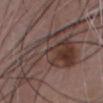biopsy status=total-body-photography surveillance lesion; no biopsy
location=the chest
lighting=white-light illumination
image=total-body-photography crop, ~15 mm field of view
subject=male, approximately 50 years of age
lesion diameter=about 11.5 mm